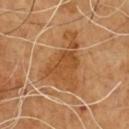Recorded during total-body skin imaging; not selected for excision or biopsy. Longest diameter approximately 7 mm. A close-up tile cropped from a whole-body skin photograph, about 15 mm across. The lesion is located on the chest. A male subject approximately 65 years of age.